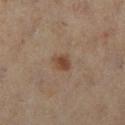follow-up — no biopsy performed (imaged during a skin exam) | acquisition — ~15 mm crop, total-body skin-cancer survey | subject — female, aged approximately 50 | site — the leg | image-analysis metrics — an area of roughly 4 mm², an eccentricity of roughly 0.65, and a symmetry-axis asymmetry near 0.15; a border-irregularity rating of about 1.5/10 and radial color variation of about 0.5; a classifier nevus-likeness of about 85/100 and lesion-presence confidence of about 100/100 | lighting — cross-polarized.